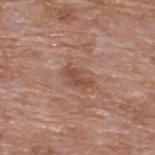The lesion was tiled from a total-body skin photograph and was not biopsied.
A 15 mm close-up extracted from a 3D total-body photography capture.
The lesion is located on the back.
The patient is a male aged around 60.